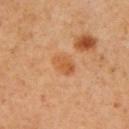Case summary:
– follow-up: catalogued during a skin exam; not biopsied
– lighting: cross-polarized illumination
– patient: male, aged 58–62
– site: the right upper arm
– image: total-body-photography crop, ~15 mm field of view
– size: ~3 mm (longest diameter)